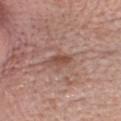This lesion was catalogued during total-body skin photography and was not selected for biopsy.
Longest diameter approximately 2.5 mm.
The lesion-visualizer software estimated a lesion area of about 3 mm² and a shape-asymmetry score of about 0.25 (0 = symmetric). And it measured internal color variation of about 1 on a 0–10 scale and a peripheral color-asymmetry measure near 0.5. The analysis additionally found an automated nevus-likeness rating near 5 out of 100.
Cropped from a total-body skin-imaging series; the visible field is about 15 mm.
The subject is a female aged 58–62.
The lesion is on the head or neck.